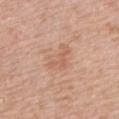Impression:
Captured during whole-body skin photography for melanoma surveillance; the lesion was not biopsied.
Clinical summary:
The lesion is on the mid back. Cropped from a total-body skin-imaging series; the visible field is about 15 mm. The total-body-photography lesion software estimated an area of roughly 7 mm², a shape eccentricity near 0.8, and two-axis asymmetry of about 0.4. The analysis additionally found a peripheral color-asymmetry measure near 0.5. The recorded lesion diameter is about 4 mm. A male patient, aged around 75.